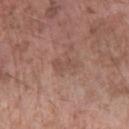| field | value |
|---|---|
| workup | no biopsy performed (imaged during a skin exam) |
| imaging modality | ~15 mm tile from a whole-body skin photo |
| patient | male, about 60 years old |
| illumination | white-light illumination |
| site | the arm |
| size | about 3 mm |